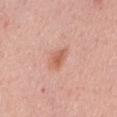Q: Was a biopsy performed?
A: no biopsy performed (imaged during a skin exam)
Q: What did automated image analysis measure?
A: a within-lesion color-variation index near 2.5/10 and radial color variation of about 1
Q: Patient demographics?
A: male, in their mid-50s
Q: How was the tile lit?
A: white-light
Q: What is the lesion's diameter?
A: ≈3 mm
Q: Lesion location?
A: the mid back
Q: How was this image acquired?
A: total-body-photography crop, ~15 mm field of view